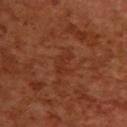Impression: Imaged during a routine full-body skin examination; the lesion was not biopsied and no histopathology is available. Acquisition and patient details: Longest diameter approximately 3 mm. On the upper back. The patient is a female approximately 55 years of age. Cropped from a total-body skin-imaging series; the visible field is about 15 mm. Imaged with cross-polarized lighting.